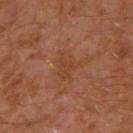Recorded during total-body skin imaging; not selected for excision or biopsy. A male subject about 30 years old. The lesion is located on the left arm. A 15 mm crop from a total-body photograph taken for skin-cancer surveillance.